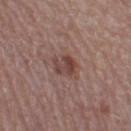Part of a total-body skin-imaging series; this lesion was reviewed on a skin check and was not flagged for biopsy. A close-up tile cropped from a whole-body skin photograph, about 15 mm across. The lesion is on the left thigh. A female subject approximately 60 years of age. Imaged with white-light lighting. The lesion's longest dimension is about 2.5 mm.